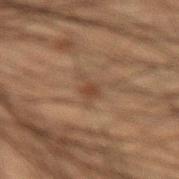Imaged during a routine full-body skin examination; the lesion was not biopsied and no histopathology is available.
The lesion is located on the left forearm.
The recorded lesion diameter is about 3 mm.
An algorithmic analysis of the crop reported about 6 CIELAB-L* units darker than the surrounding skin and a normalized border contrast of about 6.
This is a cross-polarized tile.
The subject is a male about 50 years old.
A 15 mm close-up tile from a total-body photography series done for melanoma screening.